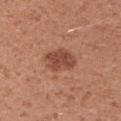follow-up = no biopsy performed (imaged during a skin exam)
image source = 15 mm crop, total-body photography
subject = male, aged 33 to 37
automated metrics = an area of roughly 8.5 mm² and a symmetry-axis asymmetry near 0.2; a detector confidence of about 100 out of 100 that the crop contains a lesion
illumination = white-light
body site = the arm
diameter = about 4 mm